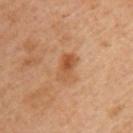Imaged during a routine full-body skin examination; the lesion was not biopsied and no histopathology is available.
The subject is a male roughly 45 years of age.
On the upper back.
A 15 mm close-up extracted from a 3D total-body photography capture.